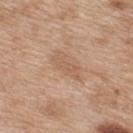- imaging modality · ~15 mm tile from a whole-body skin photo
- subject · female, approximately 40 years of age
- body site · the upper back